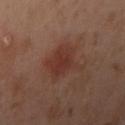follow-up=catalogued during a skin exam; not biopsied
acquisition=~15 mm tile from a whole-body skin photo
lesion size=≈4.5 mm
image-analysis metrics=a mean CIELAB color near L≈35 a*≈22 b*≈25 and a normalized border contrast of about 6.5; an automated nevus-likeness rating near 95 out of 100
lighting=cross-polarized
anatomic site=the left arm
patient=female, aged around 40